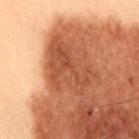notes: imaged on a skin check; not biopsied | automated lesion analysis: a lesion area of about 19 mm², a shape eccentricity near 0.75, and two-axis asymmetry of about 0.4; a border-irregularity index near 5/10, a color-variation rating of about 4/10, and a peripheral color-asymmetry measure near 1.5; a nevus-likeness score of about 5/100 and a detector confidence of about 95 out of 100 that the crop contains a lesion | size: about 6.5 mm | patient: male, aged 53–57 | acquisition: ~15 mm tile from a whole-body skin photo | body site: the back.